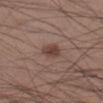Clinical impression: The lesion was photographed on a routine skin check and not biopsied; there is no pathology result. Context: A roughly 15 mm field-of-view crop from a total-body skin photograph. On the leg. Measured at roughly 2.5 mm in maximum diameter. The tile uses white-light illumination. A male patient, aged approximately 30.